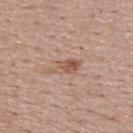biopsy status: total-body-photography surveillance lesion; no biopsy
lesion size: ≈4 mm
site: the back
acquisition: 15 mm crop, total-body photography
subject: female, in their 60s
tile lighting: white-light illumination